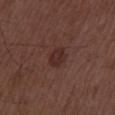The lesion was tiled from a total-body skin photograph and was not biopsied. This is a white-light tile. A close-up tile cropped from a whole-body skin photograph, about 15 mm across. A male subject, about 50 years old.